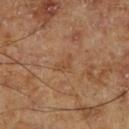Part of a total-body skin-imaging series; this lesion was reviewed on a skin check and was not flagged for biopsy. Located on the right lower leg. Longest diameter approximately 2.5 mm. A male subject aged approximately 70. The total-body-photography lesion software estimated a border-irregularity index near 4.5/10 and radial color variation of about 0.5. The software also gave an automated nevus-likeness rating near 0 out of 100 and a lesion-detection confidence of about 100/100. A 15 mm close-up tile from a total-body photography series done for melanoma screening. Imaged with cross-polarized lighting.